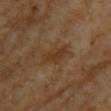anatomic site — the right upper arm
tile lighting — cross-polarized illumination
image source — ~15 mm tile from a whole-body skin photo
patient — female, about 70 years old